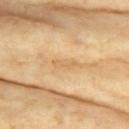Q: Is there a histopathology result?
A: no biopsy performed (imaged during a skin exam)
Q: Who is the patient?
A: female, aged around 75
Q: What kind of image is this?
A: 15 mm crop, total-body photography
Q: Where on the body is the lesion?
A: the chest